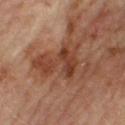Impression:
The lesion was tiled from a total-body skin photograph and was not biopsied.
Context:
The subject is a female roughly 55 years of age. On the right thigh. This image is a 15 mm lesion crop taken from a total-body photograph.